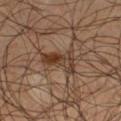{"biopsy_status": "not biopsied; imaged during a skin examination", "patient": {"sex": "male", "age_approx": 55}, "lighting": "cross-polarized", "lesion_size": {"long_diameter_mm_approx": 4.5}, "image": {"source": "total-body photography crop", "field_of_view_mm": 15}, "site": "left thigh"}